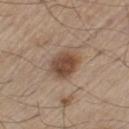workup: no biopsy performed (imaged during a skin exam) | image-analysis metrics: an average lesion color of about L≈47 a*≈17 b*≈28 (CIELAB), about 12 CIELAB-L* units darker than the surrounding skin, and a normalized lesion–skin contrast near 9; a border-irregularity index near 1/10 and peripheral color asymmetry of about 1; a lesion-detection confidence of about 100/100 | subject: male, in their 60s | diameter: about 3.5 mm | illumination: white-light illumination | imaging modality: 15 mm crop, total-body photography | location: the left thigh.